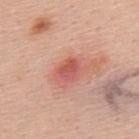Imaged during a routine full-body skin examination; the lesion was not biopsied and no histopathology is available. A close-up tile cropped from a whole-body skin photograph, about 15 mm across. From the upper back. The subject is a male aged 53–57. Longest diameter approximately 2.5 mm. The tile uses white-light illumination.